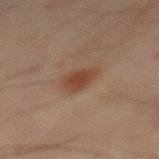Assessment:
This lesion was catalogued during total-body skin photography and was not selected for biopsy.
Context:
Located on the front of the torso. A male subject aged around 70. The tile uses cross-polarized illumination. Cropped from a total-body skin-imaging series; the visible field is about 15 mm.